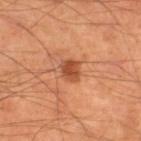Case summary:
• biopsy status · no biopsy performed (imaged during a skin exam)
• acquisition · 15 mm crop, total-body photography
• anatomic site · the right lower leg
• automated metrics · an average lesion color of about L≈50 a*≈28 b*≈38 (CIELAB), a lesion–skin lightness drop of about 12, and a normalized lesion–skin contrast near 8; an automated nevus-likeness rating near 95 out of 100
• lighting · cross-polarized illumination
• lesion size · ≈2.5 mm
• subject · male, approximately 55 years of age